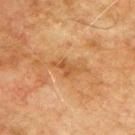Captured during whole-body skin photography for melanoma surveillance; the lesion was not biopsied. The lesion's longest dimension is about 4 mm. The tile uses cross-polarized illumination. A region of skin cropped from a whole-body photographic capture, roughly 15 mm wide. Automated tile analysis of the lesion measured an outline eccentricity of about 0.9 (0 = round, 1 = elongated). It also reported border irregularity of about 6 on a 0–10 scale, a within-lesion color-variation index near 1.5/10, and a peripheral color-asymmetry measure near 0.5. The analysis additionally found a nevus-likeness score of about 0/100 and a detector confidence of about 100 out of 100 that the crop contains a lesion. The patient is a male aged 73–77. On the upper back.